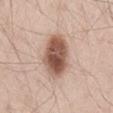Imaged during a routine full-body skin examination; the lesion was not biopsied and no histopathology is available. The lesion is located on the lower back. A male patient aged 43 to 47. Approximately 5.5 mm at its widest. Captured under white-light illumination. A lesion tile, about 15 mm wide, cut from a 3D total-body photograph.